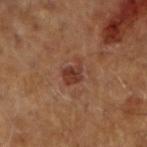The lesion was photographed on a routine skin check and not biopsied; there is no pathology result. The recorded lesion diameter is about 3 mm. The lesion is located on the right forearm. A 15 mm crop from a total-body photograph taken for skin-cancer surveillance. A male subject approximately 60 years of age.